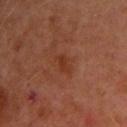Imaged during a routine full-body skin examination; the lesion was not biopsied and no histopathology is available. The lesion-visualizer software estimated a lesion area of about 3 mm², an outline eccentricity of about 0.9 (0 = round, 1 = elongated), and two-axis asymmetry of about 0.4. The software also gave a mean CIELAB color near L≈30 a*≈23 b*≈29, a lesion–skin lightness drop of about 5, and a normalized lesion–skin contrast near 6.5. The software also gave an automated nevus-likeness rating near 0 out of 100. Cropped from a whole-body photographic skin survey; the tile spans about 15 mm. Measured at roughly 2.5 mm in maximum diameter. The patient is a male aged 63 to 67. Captured under cross-polarized illumination. Located on the right upper arm.